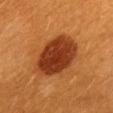Case summary:
- workup — total-body-photography surveillance lesion; no biopsy
- image source — total-body-photography crop, ~15 mm field of view
- patient — female, aged around 30
- illumination — cross-polarized
- site — the back